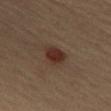| field | value |
|---|---|
| workup | total-body-photography surveillance lesion; no biopsy |
| anatomic site | the chest |
| patient | female, aged 43 to 47 |
| image source | ~15 mm tile from a whole-body skin photo |